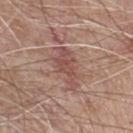Captured during whole-body skin photography for melanoma surveillance; the lesion was not biopsied. A male subject, aged 63 to 67. This image is a 15 mm lesion crop taken from a total-body photograph. The tile uses white-light illumination. The lesion's longest dimension is about 5.5 mm. The lesion is on the chest.